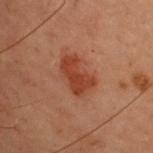Q: Is there a histopathology result?
A: no biopsy performed (imaged during a skin exam)
Q: What did automated image analysis measure?
A: a footprint of about 9 mm² and a shape eccentricity near 0.8
Q: Who is the patient?
A: female, aged 38–42
Q: Where on the body is the lesion?
A: the back
Q: What is the imaging modality?
A: ~15 mm tile from a whole-body skin photo
Q: What is the lesion's diameter?
A: about 4 mm